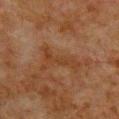Imaged during a routine full-body skin examination; the lesion was not biopsied and no histopathology is available.